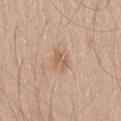Captured during whole-body skin photography for melanoma surveillance; the lesion was not biopsied. Cropped from a whole-body photographic skin survey; the tile spans about 15 mm. The lesion-visualizer software estimated about 8 CIELAB-L* units darker than the surrounding skin. And it measured a within-lesion color-variation index near 3.5/10 and a peripheral color-asymmetry measure near 1.5. From the right thigh. Measured at roughly 2.5 mm in maximum diameter. The subject is a male about 65 years old.